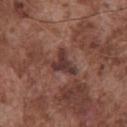Impression: No biopsy was performed on this lesion — it was imaged during a full skin examination and was not determined to be concerning. Background: Located on the chest. A male subject in their mid- to late 70s. A 15 mm close-up tile from a total-body photography series done for melanoma screening. The tile uses white-light illumination. Approximately 3.5 mm at its widest.